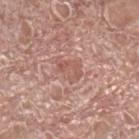Part of a total-body skin-imaging series; this lesion was reviewed on a skin check and was not flagged for biopsy.
The lesion is located on the right lower leg.
The subject is a male aged 73 to 77.
Cropped from a total-body skin-imaging series; the visible field is about 15 mm.
Longest diameter approximately 3.5 mm.